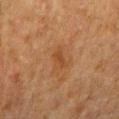• notes — no biopsy performed (imaged during a skin exam)
• lighting — cross-polarized illumination
• patient — male, aged 78 to 82
• image — total-body-photography crop, ~15 mm field of view
• site — the abdomen
• diameter — ≈3 mm